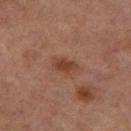Assessment: Imaged during a routine full-body skin examination; the lesion was not biopsied and no histopathology is available. Background: From the left thigh. The recorded lesion diameter is about 3 mm. A female subject, roughly 60 years of age. This is a cross-polarized tile. Cropped from a total-body skin-imaging series; the visible field is about 15 mm.